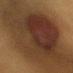Captured under cross-polarized illumination. A roughly 15 mm field-of-view crop from a total-body skin photograph. A female subject, aged 38–42. Located on the chest.